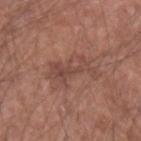Notes:
• automated metrics · a lesion color around L≈46 a*≈21 b*≈25 in CIELAB, about 7 CIELAB-L* units darker than the surrounding skin, and a lesion-to-skin contrast of about 5.5 (normalized; higher = more distinct); border irregularity of about 8.5 on a 0–10 scale, a color-variation rating of about 1.5/10, and peripheral color asymmetry of about 0.5; lesion-presence confidence of about 100/100
• body site · the left forearm
• acquisition · 15 mm crop, total-body photography
• diameter · ≈5 mm
• subject · male, aged 53–57
• lighting · white-light